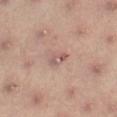{"biopsy_status": "not biopsied; imaged during a skin examination", "patient": {"sex": "male", "age_approx": 55}, "image": {"source": "total-body photography crop", "field_of_view_mm": 15}, "lighting": "cross-polarized", "site": "right thigh"}